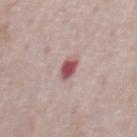<lesion>
<biopsy_status>not biopsied; imaged during a skin examination</biopsy_status>
<image>
  <source>total-body photography crop</source>
  <field_of_view_mm>15</field_of_view_mm>
</image>
<site>chest</site>
<patient>
  <sex>male</sex>
  <age_approx>75</age_approx>
</patient>
</lesion>